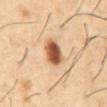<case>
  <biopsy_status>not biopsied; imaged during a skin examination</biopsy_status>
  <lighting>cross-polarized</lighting>
  <site>front of the torso</site>
  <lesion_size>
    <long_diameter_mm_approx>3.5</long_diameter_mm_approx>
  </lesion_size>
  <patient>
    <sex>male</sex>
    <age_approx>55</age_approx>
  </patient>
  <image>
    <source>total-body photography crop</source>
    <field_of_view_mm>15</field_of_view_mm>
  </image>
</case>